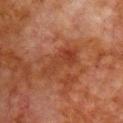  biopsy_status: not biopsied; imaged during a skin examination
  patient:
    sex: male
    age_approx: 80
  image:
    source: total-body photography crop
    field_of_view_mm: 15
  site: front of the torso
  lighting: cross-polarized